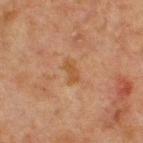Background: The recorded lesion diameter is about 2.5 mm. From the chest. A roughly 15 mm field-of-view crop from a total-body skin photograph. The tile uses cross-polarized illumination. The subject is a male aged approximately 65.